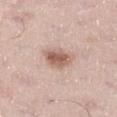The lesion was tiled from a total-body skin photograph and was not biopsied. A male patient, aged approximately 55. On the left thigh. Cropped from a total-body skin-imaging series; the visible field is about 15 mm.